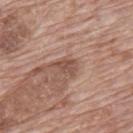Findings:
• follow-up — imaged on a skin check; not biopsied
• anatomic site — the upper back
• lighting — white-light
• image source — total-body-photography crop, ~15 mm field of view
• patient — male, roughly 70 years of age
• TBP lesion metrics — a lesion area of about 4 mm² and an outline eccentricity of about 0.75 (0 = round, 1 = elongated); a border-irregularity rating of about 3.5/10 and a within-lesion color-variation index near 2.5/10; an automated nevus-likeness rating near 10 out of 100 and a lesion-detection confidence of about 100/100